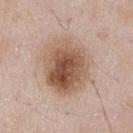{
  "biopsy_status": "not biopsied; imaged during a skin examination",
  "lesion_size": {
    "long_diameter_mm_approx": 6.5
  },
  "image": {
    "source": "total-body photography crop",
    "field_of_view_mm": 15
  },
  "lighting": "white-light",
  "patient": {
    "sex": "male",
    "age_approx": 55
  },
  "site": "chest",
  "automated_metrics": {
    "cielab_L": 55,
    "cielab_a": 19,
    "cielab_b": 28,
    "border_irregularity_0_10": 1.5,
    "color_variation_0_10": 8.5,
    "peripheral_color_asymmetry": 3.0
  }
}